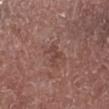Part of a total-body skin-imaging series; this lesion was reviewed on a skin check and was not flagged for biopsy. Cropped from a whole-body photographic skin survey; the tile spans about 15 mm. From the left lower leg. The patient is a male aged around 55. Captured under white-light illumination. The lesion-visualizer software estimated a mean CIELAB color near L≈43 a*≈21 b*≈23, a lesion–skin lightness drop of about 7, and a normalized border contrast of about 5.5. The software also gave internal color variation of about 0 on a 0–10 scale and a peripheral color-asymmetry measure near 0. Longest diameter approximately 2.5 mm.